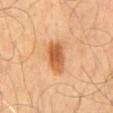{"biopsy_status": "not biopsied; imaged during a skin examination", "image": {"source": "total-body photography crop", "field_of_view_mm": 15}, "patient": {"sex": "male", "age_approx": 55}, "automated_metrics": {"area_mm2_approx": 8.5, "eccentricity": 0.8, "shape_asymmetry": 0.2}, "lesion_size": {"long_diameter_mm_approx": 4.0}, "lighting": "cross-polarized", "site": "mid back"}